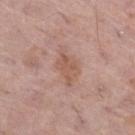patient:
  sex: female
  age_approx: 60
image:
  source: total-body photography crop
  field_of_view_mm: 15
lighting: white-light
lesion_size:
  long_diameter_mm_approx: 4.0
site: right thigh